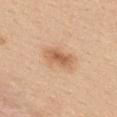A female patient approximately 45 years of age.
The recorded lesion diameter is about 4 mm.
Captured under white-light illumination.
A 15 mm crop from a total-body photograph taken for skin-cancer surveillance.
The lesion is located on the mid back.
Automated image analysis of the tile measured a border-irregularity index near 3/10, a color-variation rating of about 4/10, and radial color variation of about 1. The software also gave a nevus-likeness score of about 90/100 and a lesion-detection confidence of about 100/100.